A male subject aged around 70. This image is a 15 mm lesion crop taken from a total-body photograph. The recorded lesion diameter is about 7 mm. Located on the upper back — 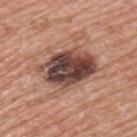histopathologic diagnosis=a dysplastic (Clark) nevus.